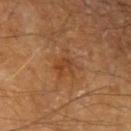The lesion was photographed on a routine skin check and not biopsied; there is no pathology result.
The total-body-photography lesion software estimated an average lesion color of about L≈42 a*≈22 b*≈35 (CIELAB), a lesion–skin lightness drop of about 7, and a normalized lesion–skin contrast near 5.5. The analysis additionally found a nevus-likeness score of about 10/100 and a detector confidence of about 100 out of 100 that the crop contains a lesion.
From the left forearm.
A region of skin cropped from a whole-body photographic capture, roughly 15 mm wide.
A male subject in their 70s.
The lesion's longest dimension is about 4 mm.